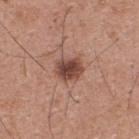biopsy_status: not biopsied; imaged during a skin examination
image:
  source: total-body photography crop
  field_of_view_mm: 15
automated_metrics:
  eccentricity: 0.55
  shape_asymmetry: 0.2
lighting: white-light
patient:
  sex: male
  age_approx: 30
lesion_size:
  long_diameter_mm_approx: 3.5
site: upper back